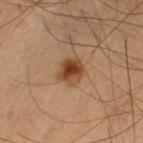Notes:
- notes · total-body-photography surveillance lesion; no biopsy
- imaging modality · total-body-photography crop, ~15 mm field of view
- patient · male, roughly 60 years of age
- body site · the leg
- image-analysis metrics · a lesion area of about 7.5 mm²; an average lesion color of about L≈39 a*≈19 b*≈30 (CIELAB), roughly 11 lightness units darker than nearby skin, and a lesion-to-skin contrast of about 10 (normalized; higher = more distinct); a classifier nevus-likeness of about 100/100
- size · ~3.5 mm (longest diameter)